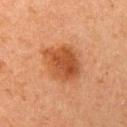Q: Is there a histopathology result?
A: catalogued during a skin exam; not biopsied
Q: Illumination type?
A: cross-polarized
Q: Patient demographics?
A: female, roughly 60 years of age
Q: What kind of image is this?
A: ~15 mm crop, total-body skin-cancer survey
Q: Where on the body is the lesion?
A: the left upper arm